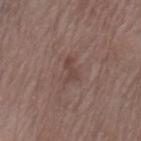Clinical impression:
The lesion was photographed on a routine skin check and not biopsied; there is no pathology result.
Clinical summary:
A female subject aged approximately 70. The lesion-visualizer software estimated a footprint of about 2.5 mm², a shape eccentricity near 0.9, and a symmetry-axis asymmetry near 0.5. It also reported a lesion color around L≈42 a*≈18 b*≈22 in CIELAB and a lesion–skin lightness drop of about 7. And it measured a within-lesion color-variation index near 0/10. The software also gave an automated nevus-likeness rating near 0 out of 100. Cropped from a total-body skin-imaging series; the visible field is about 15 mm. Captured under white-light illumination. Measured at roughly 2.5 mm in maximum diameter. Located on the left thigh.